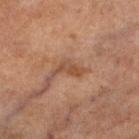No biopsy was performed on this lesion — it was imaged during a full skin examination and was not determined to be concerning. The subject is a female aged 58–62. The lesion's longest dimension is about 3.5 mm. A roughly 15 mm field-of-view crop from a total-body skin photograph. An algorithmic analysis of the crop reported border irregularity of about 5.5 on a 0–10 scale. The tile uses cross-polarized illumination. Located on the left lower leg.